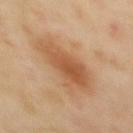<case>
  <lesion_size>
    <long_diameter_mm_approx>6.0</long_diameter_mm_approx>
  </lesion_size>
  <lighting>cross-polarized</lighting>
  <image>
    <source>total-body photography crop</source>
    <field_of_view_mm>15</field_of_view_mm>
  </image>
  <automated_metrics>
    <area_mm2_approx>19.0</area_mm2_approx>
    <eccentricity>0.75</eccentricity>
    <shape_asymmetry>0.3</shape_asymmetry>
    <cielab_L>57</cielab_L>
    <cielab_a>22</cielab_a>
    <cielab_b>37</cielab_b>
    <vs_skin_darker_L>10.0</vs_skin_darker_L>
    <vs_skin_contrast_norm>7.0</vs_skin_contrast_norm>
    <border_irregularity_0_10>4.0</border_irregularity_0_10>
    <color_variation_0_10>4.0</color_variation_0_10>
    <peripheral_color_asymmetry>1.0</peripheral_color_asymmetry>
  </automated_metrics>
  <patient>
    <sex>female</sex>
    <age_approx>45</age_approx>
  </patient>
  <site>upper back</site>
</case>